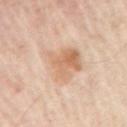{
  "biopsy_status": "not biopsied; imaged during a skin examination"
}